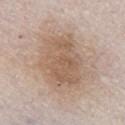biopsy status — no biopsy performed (imaged during a skin exam)
anatomic site — the right upper arm
acquisition — ~15 mm crop, total-body skin-cancer survey
patient — male, aged 63–67
lesion size — ≈7 mm
TBP lesion metrics — a footprint of about 26 mm²; a mean CIELAB color near L≈58 a*≈16 b*≈29 and a lesion-to-skin contrast of about 6.5 (normalized; higher = more distinct); border irregularity of about 2.5 on a 0–10 scale, a color-variation rating of about 4/10, and radial color variation of about 1.5; a classifier nevus-likeness of about 35/100 and a lesion-detection confidence of about 100/100